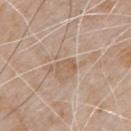A male subject roughly 80 years of age. A lesion tile, about 15 mm wide, cut from a 3D total-body photograph. The lesion is on the chest.